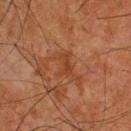Captured during whole-body skin photography for melanoma surveillance; the lesion was not biopsied.
Imaged with cross-polarized lighting.
A roughly 15 mm field-of-view crop from a total-body skin photograph.
The subject is a male about 65 years old.
Approximately 2.5 mm at its widest.
The lesion is on the upper back.
The lesion-visualizer software estimated an area of roughly 3.5 mm² and two-axis asymmetry of about 0.5. And it measured an average lesion color of about L≈32 a*≈21 b*≈30 (CIELAB), roughly 5 lightness units darker than nearby skin, and a lesion-to-skin contrast of about 5.5 (normalized; higher = more distinct). The analysis additionally found a nevus-likeness score of about 0/100.